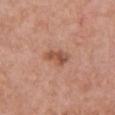Case summary:
• workup: catalogued during a skin exam; not biopsied
• subject: female, aged approximately 60
• anatomic site: the chest
• image source: 15 mm crop, total-body photography